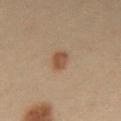  biopsy_status: not biopsied; imaged during a skin examination
  patient:
    sex: female
    age_approx: 30
  image:
    source: total-body photography crop
    field_of_view_mm: 15
  lighting: cross-polarized
  site: abdomen